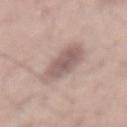follow-up: imaged on a skin check; not biopsied
anatomic site: the mid back
automated metrics: a lesion area of about 14 mm², an eccentricity of roughly 0.85, and two-axis asymmetry of about 0.25; a lesion color around L≈58 a*≈16 b*≈21 in CIELAB, about 11 CIELAB-L* units darker than the surrounding skin, and a lesion-to-skin contrast of about 7.5 (normalized; higher = more distinct)
image: 15 mm crop, total-body photography
patient: male, aged 53–57